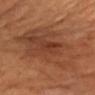Case summary:
- biopsy status: no biopsy performed (imaged during a skin exam)
- image: ~15 mm tile from a whole-body skin photo
- automated metrics: a mean CIELAB color near L≈40 a*≈22 b*≈31, a lesion–skin lightness drop of about 7, and a normalized lesion–skin contrast near 6; a nevus-likeness score of about 5/100
- size: about 9.5 mm
- tile lighting: cross-polarized
- patient: male, aged 68–72
- site: the chest